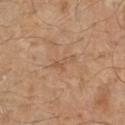Captured during whole-body skin photography for melanoma surveillance; the lesion was not biopsied. A male subject, about 70 years old. The lesion-visualizer software estimated an eccentricity of roughly 0.95 and a shape-asymmetry score of about 0.4 (0 = symmetric). Cropped from a whole-body photographic skin survey; the tile spans about 15 mm. On the chest. This is a white-light tile. Measured at roughly 3 mm in maximum diameter.